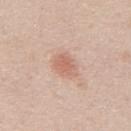No biopsy was performed on this lesion — it was imaged during a full skin examination and was not determined to be concerning. Located on the mid back. A male patient approximately 50 years of age. Automated image analysis of the tile measured an area of roughly 6.5 mm², a shape eccentricity near 0.7, and two-axis asymmetry of about 0.25. The software also gave a border-irregularity rating of about 2/10, internal color variation of about 2.5 on a 0–10 scale, and peripheral color asymmetry of about 1. It also reported a classifier nevus-likeness of about 70/100 and a detector confidence of about 100 out of 100 that the crop contains a lesion. A roughly 15 mm field-of-view crop from a total-body skin photograph.